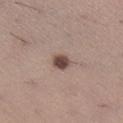biopsy_status: not biopsied; imaged during a skin examination
automated_metrics:
  cielab_L: 45
  cielab_a: 17
  cielab_b: 21
  vs_skin_darker_L: 16.0
  vs_skin_contrast_norm: 11.5
lighting: white-light
lesion_size:
  long_diameter_mm_approx: 2.0
site: right lower leg
patient:
  sex: female
  age_approx: 40
image:
  source: total-body photography crop
  field_of_view_mm: 15